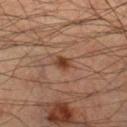A region of skin cropped from a whole-body photographic capture, roughly 15 mm wide.
A male subject aged approximately 45.
The lesion is on the right lower leg.